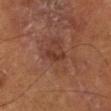Impression: Recorded during total-body skin imaging; not selected for excision or biopsy. Acquisition and patient details: The lesion is on the left lower leg. Imaged with cross-polarized lighting. A region of skin cropped from a whole-body photographic capture, roughly 15 mm wide. A male patient approximately 55 years of age. The lesion-visualizer software estimated a footprint of about 3 mm² and a symmetry-axis asymmetry near 0.4. About 3 mm across.